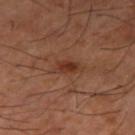follow-up: no biopsy performed (imaged during a skin exam); patient: male, approximately 65 years of age; body site: the right thigh; acquisition: 15 mm crop, total-body photography.